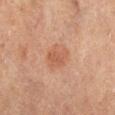Assessment:
This lesion was catalogued during total-body skin photography and was not selected for biopsy.
Acquisition and patient details:
The tile uses cross-polarized illumination. The lesion is on the right lower leg. The subject is a male in their mid- to late 60s. A close-up tile cropped from a whole-body skin photograph, about 15 mm across.